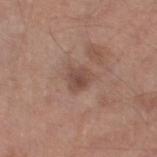Background:
The total-body-photography lesion software estimated a footprint of about 5 mm², a shape eccentricity near 0.5, and a shape-asymmetry score of about 0.3 (0 = symmetric). And it measured a mean CIELAB color near L≈47 a*≈18 b*≈25, roughly 9 lightness units darker than nearby skin, and a normalized border contrast of about 6.5. The analysis additionally found a classifier nevus-likeness of about 0/100 and a detector confidence of about 100 out of 100 that the crop contains a lesion. On the leg. The subject is a male approximately 65 years of age. Cropped from a whole-body photographic skin survey; the tile spans about 15 mm. The lesion's longest dimension is about 2.5 mm.